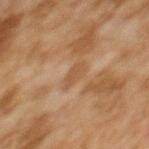Clinical impression:
The lesion was tiled from a total-body skin photograph and was not biopsied.
Image and clinical context:
The lesion is on the upper back. The patient is a female about 60 years old. Approximately 3 mm at its widest. A lesion tile, about 15 mm wide, cut from a 3D total-body photograph. This is a cross-polarized tile.